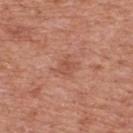The lesion was photographed on a routine skin check and not biopsied; there is no pathology result.